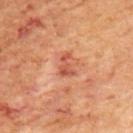Clinical impression: The lesion was tiled from a total-body skin photograph and was not biopsied. Acquisition and patient details: The lesion is on the left upper arm. The recorded lesion diameter is about 2.5 mm. A region of skin cropped from a whole-body photographic capture, roughly 15 mm wide. Imaged with cross-polarized lighting. Automated image analysis of the tile measured an area of roughly 4 mm². The software also gave a classifier nevus-likeness of about 0/100 and lesion-presence confidence of about 100/100. A female subject, roughly 50 years of age.